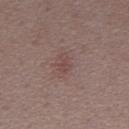  biopsy_status: not biopsied; imaged during a skin examination
  patient:
    sex: male
    age_approx: 55
  site: abdomen
  automated_metrics:
    area_mm2_approx: 3.0
    eccentricity: 0.85
    shape_asymmetry: 0.35
    vs_skin_darker_L: 6.0
    vs_skin_contrast_norm: 5.0
    border_irregularity_0_10: 3.5
    color_variation_0_10: 0.5
    peripheral_color_asymmetry: 0.0
  image:
    source: total-body photography crop
    field_of_view_mm: 15